A 15 mm close-up tile from a total-body photography series done for melanoma screening · the subject is a female aged around 25 · from the leg · captured under cross-polarized illumination.
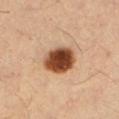Q: What did the biopsy show?
A: a melanoma in situ — a malignant lesion